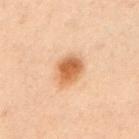notes: catalogued during a skin exam; not biopsied | subject: male, about 55 years old | lesion diameter: about 4 mm | imaging modality: ~15 mm crop, total-body skin-cancer survey | location: the left upper arm.